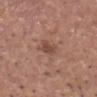This lesion was catalogued during total-body skin photography and was not selected for biopsy. A male patient aged 63 to 67. Approximately 2.5 mm at its widest. Imaged with white-light lighting. On the head or neck. Cropped from a whole-body photographic skin survey; the tile spans about 15 mm.